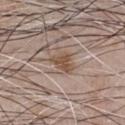{"biopsy_status": "not biopsied; imaged during a skin examination", "patient": {"sex": "male", "age_approx": 50}, "automated_metrics": {"border_irregularity_0_10": 4.5, "peripheral_color_asymmetry": 1.0}, "lighting": "white-light", "site": "chest", "image": {"source": "total-body photography crop", "field_of_view_mm": 15}}